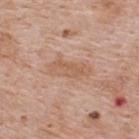This lesion was catalogued during total-body skin photography and was not selected for biopsy.
A 15 mm close-up extracted from a 3D total-body photography capture.
The recorded lesion diameter is about 4.5 mm.
A male subject, in their mid-60s.
Automated image analysis of the tile measured a mean CIELAB color near L≈58 a*≈20 b*≈31, a lesion–skin lightness drop of about 8, and a normalized border contrast of about 6. And it measured border irregularity of about 3.5 on a 0–10 scale, internal color variation of about 2 on a 0–10 scale, and a peripheral color-asymmetry measure near 1.
The lesion is located on the back.
Imaged with white-light lighting.